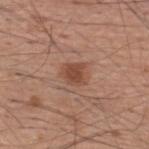{"biopsy_status": "not biopsied; imaged during a skin examination", "image": {"source": "total-body photography crop", "field_of_view_mm": 15}, "site": "upper back", "lighting": "white-light", "lesion_size": {"long_diameter_mm_approx": 3.0}, "automated_metrics": {"cielab_L": 47, "cielab_a": 22, "cielab_b": 29, "vs_skin_darker_L": 9.0, "vs_skin_contrast_norm": 7.5}, "patient": {"sex": "male", "age_approx": 60}}